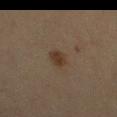Case summary:
• notes — imaged on a skin check; not biopsied
• acquisition — total-body-photography crop, ~15 mm field of view
• tile lighting — cross-polarized illumination
• subject — female, in their 70s
• body site — the left thigh
• lesion size — ≈2.5 mm